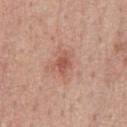  biopsy_status: not biopsied; imaged during a skin examination
  site: front of the torso
  lesion_size:
    long_diameter_mm_approx: 3.0
  patient:
    sex: male
    age_approx: 50
  automated_metrics:
    area_mm2_approx: 4.0
    vs_skin_contrast_norm: 7.0
    border_irregularity_0_10: 3.0
    color_variation_0_10: 2.5
    peripheral_color_asymmetry: 1.0
  image:
    source: total-body photography crop
    field_of_view_mm: 15